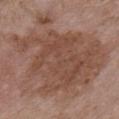follow-up — catalogued during a skin exam; not biopsied | lesion diameter — ≈13 mm | tile lighting — white-light illumination | anatomic site — the chest | TBP lesion metrics — a footprint of about 80 mm² and an outline eccentricity of about 0.55 (0 = round, 1 = elongated); roughly 9 lightness units darker than nearby skin and a normalized lesion–skin contrast near 6.5; a detector confidence of about 100 out of 100 that the crop contains a lesion | image source — ~15 mm tile from a whole-body skin photo | subject — male, roughly 50 years of age.